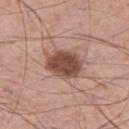Imaged during a routine full-body skin examination; the lesion was not biopsied and no histopathology is available. Located on the left thigh. A region of skin cropped from a whole-body photographic capture, roughly 15 mm wide. This is a white-light tile. A male subject, aged 53–57. Approximately 4.5 mm at its widest.